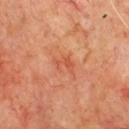| feature | finding |
|---|---|
| workup | total-body-photography surveillance lesion; no biopsy |
| location | the chest |
| subject | male, approximately 70 years of age |
| illumination | cross-polarized |
| image source | ~15 mm crop, total-body skin-cancer survey |